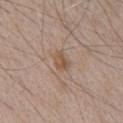Acquisition and patient details: About 2.5 mm across. The lesion is on the front of the torso. A 15 mm close-up tile from a total-body photography series done for melanoma screening. The patient is a male roughly 65 years of age. The tile uses white-light illumination.